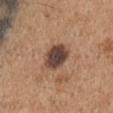Context: Cropped from a whole-body photographic skin survey; the tile spans about 15 mm. A male subject in their mid-60s. This is a white-light tile. On the right upper arm. About 4 mm across.